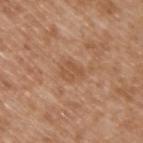TBP lesion metrics = a color-variation rating of about 2/10 and peripheral color asymmetry of about 0.5; an automated nevus-likeness rating near 0 out of 100 and a detector confidence of about 100 out of 100 that the crop contains a lesion
tile lighting = white-light illumination
image source = total-body-photography crop, ~15 mm field of view
anatomic site = the upper back
subject = male, roughly 65 years of age